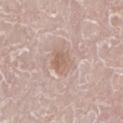Assessment: The lesion was photographed on a routine skin check and not biopsied; there is no pathology result. Background: A roughly 15 mm field-of-view crop from a total-body skin photograph. Located on the left lower leg. The subject is a male aged around 80. Measured at roughly 3 mm in maximum diameter.